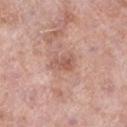notes: catalogued during a skin exam; not biopsied | site: the left lower leg | automated metrics: an area of roughly 4 mm², an outline eccentricity of about 0.85 (0 = round, 1 = elongated), and a shape-asymmetry score of about 0.3 (0 = symmetric); an average lesion color of about L≈56 a*≈22 b*≈28 (CIELAB), roughly 9 lightness units darker than nearby skin, and a lesion-to-skin contrast of about 6.5 (normalized; higher = more distinct); a border-irregularity index near 4/10 | lesion size: about 3 mm | tile lighting: white-light illumination | image source: 15 mm crop, total-body photography | patient: male, aged 73 to 77.